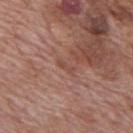biopsy_status: not biopsied; imaged during a skin examination
patient:
  sex: male
  age_approx: 70
image:
  source: total-body photography crop
  field_of_view_mm: 15
site: mid back